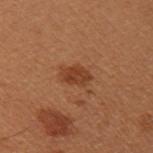<tbp_lesion>
  <biopsy_status>not biopsied; imaged during a skin examination</biopsy_status>
  <site>left upper arm</site>
  <patient>
    <sex>female</sex>
    <age_approx>50</age_approx>
  </patient>
  <image>
    <source>total-body photography crop</source>
    <field_of_view_mm>15</field_of_view_mm>
  </image>
  <lighting>cross-polarized</lighting>
  <lesion_size>
    <long_diameter_mm_approx>3.0</long_diameter_mm_approx>
  </lesion_size>
</tbp_lesion>